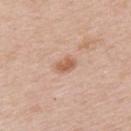Clinical impression: Imaged during a routine full-body skin examination; the lesion was not biopsied and no histopathology is available. Acquisition and patient details: Measured at roughly 2.5 mm in maximum diameter. A female patient, in their mid-40s. Automated image analysis of the tile measured an eccentricity of roughly 0.7. The software also gave an average lesion color of about L≈60 a*≈21 b*≈32 (CIELAB). A lesion tile, about 15 mm wide, cut from a 3D total-body photograph. Captured under white-light illumination. Located on the back.